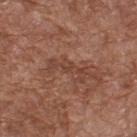  automated_metrics:
    eccentricity: 0.95
    lesion_detection_confidence_0_100: 80
  image:
    source: total-body photography crop
    field_of_view_mm: 15
  lighting: white-light
  patient:
    sex: male
    age_approx: 75
  lesion_size:
    long_diameter_mm_approx: 4.5
  site: upper back